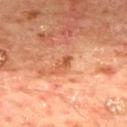Impression:
Recorded during total-body skin imaging; not selected for excision or biopsy.
Background:
A 15 mm close-up extracted from a 3D total-body photography capture. The patient is a male aged approximately 65. Located on the mid back. The lesion-visualizer software estimated a mean CIELAB color near L≈56 a*≈29 b*≈40, roughly 9 lightness units darker than nearby skin, and a normalized lesion–skin contrast near 6.5. And it measured border irregularity of about 4 on a 0–10 scale, a within-lesion color-variation index near 0.5/10, and peripheral color asymmetry of about 0.